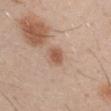biopsy status: no biopsy performed (imaged during a skin exam)